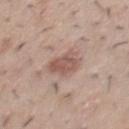{
  "biopsy_status": "not biopsied; imaged during a skin examination",
  "lesion_size": {
    "long_diameter_mm_approx": 3.5
  },
  "image": {
    "source": "total-body photography crop",
    "field_of_view_mm": 15
  },
  "site": "chest",
  "patient": {
    "sex": "male",
    "age_approx": 30
  },
  "lighting": "white-light"
}